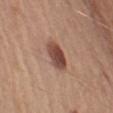Measured at roughly 3.5 mm in maximum diameter. Imaged with white-light lighting. A male patient, about 65 years old. On the left upper arm. A 15 mm close-up extracted from a 3D total-body photography capture.